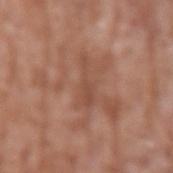Captured during whole-body skin photography for melanoma surveillance; the lesion was not biopsied. A male patient aged 58–62. The lesion is on the left upper arm. A 15 mm close-up tile from a total-body photography series done for melanoma screening.